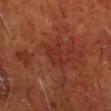| key | value |
|---|---|
| subject | male, aged around 60 |
| anatomic site | the arm |
| TBP lesion metrics | a mean CIELAB color near L≈30 a*≈26 b*≈28 and a lesion–skin lightness drop of about 5; a detector confidence of about 100 out of 100 that the crop contains a lesion |
| image | 15 mm crop, total-body photography |
| lighting | cross-polarized illumination |
| lesion size | ~3 mm (longest diameter) |